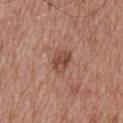The lesion was photographed on a routine skin check and not biopsied; there is no pathology result.
Captured under white-light illumination.
The lesion is located on the upper back.
A male patient aged 63–67.
A lesion tile, about 15 mm wide, cut from a 3D total-body photograph.
Longest diameter approximately 3 mm.
The total-body-photography lesion software estimated a normalized border contrast of about 7. It also reported a border-irregularity rating of about 2/10 and a peripheral color-asymmetry measure near 2.